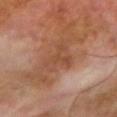  biopsy_status: not biopsied; imaged during a skin examination
  site: right upper arm
  automated_metrics:
    border_irregularity_0_10: 9.5
    color_variation_0_10: 2.5
    peripheral_color_asymmetry: 1.0
    nevus_likeness_0_100: 0
  image:
    source: total-body photography crop
    field_of_view_mm: 15
  patient:
    sex: male
    age_approx: 70
  lighting: cross-polarized
  lesion_size:
    long_diameter_mm_approx: 4.5